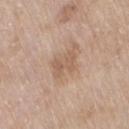A region of skin cropped from a whole-body photographic capture, roughly 15 mm wide. Imaged with white-light lighting. A female patient, in their mid- to late 70s. On the leg.